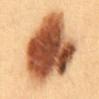* workup · no biopsy performed (imaged during a skin exam)
* size · about 9.5 mm
* illumination · cross-polarized illumination
* body site · the chest
* image-analysis metrics · a footprint of about 60 mm², an outline eccentricity of about 0.45 (0 = round, 1 = elongated), and two-axis asymmetry of about 0.25; a border-irregularity index near 2.5/10, a within-lesion color-variation index near 9.5/10, and a peripheral color-asymmetry measure near 3; an automated nevus-likeness rating near 85 out of 100 and a detector confidence of about 100 out of 100 that the crop contains a lesion
* imaging modality · 15 mm crop, total-body photography
* patient · female, about 30 years old